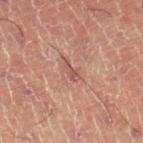notes: no biopsy performed (imaged during a skin exam); image source: 15 mm crop, total-body photography; patient: male, approximately 60 years of age.